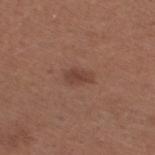workup = no biopsy performed (imaged during a skin exam) | lighting = white-light illumination | image = 15 mm crop, total-body photography | location = the right thigh | TBP lesion metrics = a lesion area of about 3.5 mm², an eccentricity of roughly 0.85, and two-axis asymmetry of about 0.25; a lesion color around L≈41 a*≈21 b*≈25 in CIELAB, a lesion–skin lightness drop of about 8, and a normalized border contrast of about 6.5; a nevus-likeness score of about 75/100 and a detector confidence of about 100 out of 100 that the crop contains a lesion | patient = female, aged 53 to 57.